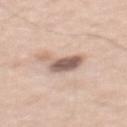Impression:
No biopsy was performed on this lesion — it was imaged during a full skin examination and was not determined to be concerning.
Clinical summary:
Automated image analysis of the tile measured an area of roughly 8 mm² and a symmetry-axis asymmetry near 0.5. And it measured an average lesion color of about L≈59 a*≈17 b*≈25 (CIELAB), a lesion–skin lightness drop of about 16, and a normalized border contrast of about 9.5. And it measured a border-irregularity index near 5.5/10, a within-lesion color-variation index near 3.5/10, and radial color variation of about 1. The analysis additionally found a classifier nevus-likeness of about 30/100 and a lesion-detection confidence of about 100/100. The lesion is on the mid back. A male patient, in their 60s. A region of skin cropped from a whole-body photographic capture, roughly 15 mm wide. The tile uses white-light illumination. About 4 mm across.